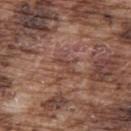Impression:
The lesion was photographed on a routine skin check and not biopsied; there is no pathology result.
Context:
This is a white-light tile. The lesion is on the upper back. The subject is a male roughly 75 years of age. A 15 mm crop from a total-body photograph taken for skin-cancer surveillance.